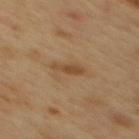Recorded during total-body skin imaging; not selected for excision or biopsy.
An algorithmic analysis of the crop reported a shape-asymmetry score of about 0.35 (0 = symmetric).
Captured under cross-polarized illumination.
The lesion is located on the mid back.
Longest diameter approximately 3 mm.
The patient is a male aged approximately 50.
This image is a 15 mm lesion crop taken from a total-body photograph.